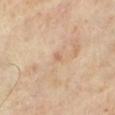Background: The total-body-photography lesion software estimated a lesion area of about 1 mm², an outline eccentricity of about 0.75 (0 = round, 1 = elongated), and two-axis asymmetry of about 0.3. And it measured a border-irregularity rating of about 2.5/10 and peripheral color asymmetry of about 0. Located on the left lower leg. The tile uses cross-polarized illumination. This image is a 15 mm lesion crop taken from a total-body photograph. Measured at roughly 1 mm in maximum diameter. A female patient aged around 70.